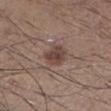| key | value |
|---|---|
| notes | catalogued during a skin exam; not biopsied |
| image | 15 mm crop, total-body photography |
| illumination | white-light illumination |
| subject | male, aged approximately 35 |
| automated metrics | a mean CIELAB color near L≈42 a*≈17 b*≈21; a border-irregularity index near 2/10, internal color variation of about 3 on a 0–10 scale, and radial color variation of about 1 |
| anatomic site | the left lower leg |